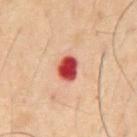The lesion was photographed on a routine skin check and not biopsied; there is no pathology result.
From the abdomen.
A 15 mm crop from a total-body photograph taken for skin-cancer surveillance.
The subject is a male about 50 years old.
Measured at roughly 3 mm in maximum diameter.
The lesion-visualizer software estimated a border-irregularity index near 1.5/10 and a color-variation rating of about 3.5/10.